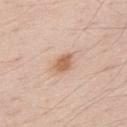<tbp_lesion>
<biopsy_status>not biopsied; imaged during a skin examination</biopsy_status>
<image>
  <source>total-body photography crop</source>
  <field_of_view_mm>15</field_of_view_mm>
</image>
<automated_metrics>
  <nevus_likeness_0_100>85</nevus_likeness_0_100>
  <lesion_detection_confidence_0_100>100</lesion_detection_confidence_0_100>
</automated_metrics>
<site>back</site>
<lesion_size>
  <long_diameter_mm_approx>2.5</long_diameter_mm_approx>
</lesion_size>
<lighting>white-light</lighting>
<patient>
  <sex>male</sex>
  <age_approx>70</age_approx>
</patient>
</tbp_lesion>